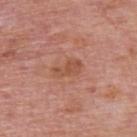No biopsy was performed on this lesion — it was imaged during a full skin examination and was not determined to be concerning.
The lesion is located on the upper back.
Automated image analysis of the tile measured a footprint of about 5.5 mm², an outline eccentricity of about 0.85 (0 = round, 1 = elongated), and a shape-asymmetry score of about 0.3 (0 = symmetric). It also reported a lesion color around L≈52 a*≈24 b*≈32 in CIELAB and roughly 7 lightness units darker than nearby skin. And it measured a border-irregularity rating of about 3.5/10, a within-lesion color-variation index near 2/10, and a peripheral color-asymmetry measure near 0.5. The software also gave a detector confidence of about 100 out of 100 that the crop contains a lesion.
The tile uses white-light illumination.
Cropped from a total-body skin-imaging series; the visible field is about 15 mm.
The subject is a male roughly 75 years of age.
The recorded lesion diameter is about 3.5 mm.